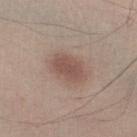biopsy status: total-body-photography surveillance lesion; no biopsy
illumination: white-light illumination
body site: the right lower leg
image-analysis metrics: an area of roughly 8.5 mm², an outline eccentricity of about 0.8 (0 = round, 1 = elongated), and a symmetry-axis asymmetry near 0.2; a normalized lesion–skin contrast near 7; border irregularity of about 2 on a 0–10 scale, a color-variation rating of about 2/10, and a peripheral color-asymmetry measure near 0.5
subject: male, aged 43 to 47
lesion size: ~4 mm (longest diameter)
acquisition: total-body-photography crop, ~15 mm field of view